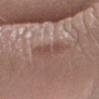{
  "biopsy_status": "not biopsied; imaged during a skin examination",
  "patient": {
    "sex": "female",
    "age_approx": 50
  },
  "image": {
    "source": "total-body photography crop",
    "field_of_view_mm": 15
  },
  "lesion_size": {
    "long_diameter_mm_approx": 4.5
  },
  "lighting": "white-light",
  "site": "arm"
}